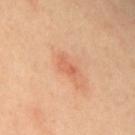Notes:
* notes — no biopsy performed (imaged during a skin exam)
* location — the upper back
* patient — female, aged 38 to 42
* image source — total-body-photography crop, ~15 mm field of view